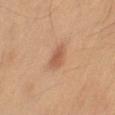notes: catalogued during a skin exam; not biopsied | body site: the right thigh | imaging modality: ~15 mm crop, total-body skin-cancer survey | lesion diameter: about 3.5 mm | subject: female, approximately 60 years of age | illumination: cross-polarized.